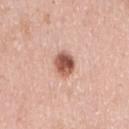<case>
  <biopsy_status>not biopsied; imaged during a skin examination</biopsy_status>
  <lighting>white-light</lighting>
  <site>arm</site>
  <image>
    <source>total-body photography crop</source>
    <field_of_view_mm>15</field_of_view_mm>
  </image>
  <patient>
    <sex>female</sex>
    <age_approx>50</age_approx>
  </patient>
  <lesion_size>
    <long_diameter_mm_approx>2.5</long_diameter_mm_approx>
  </lesion_size>
</case>